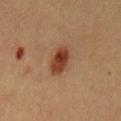biopsy status — imaged on a skin check; not biopsied
patient — female, approximately 40 years of age
image — 15 mm crop, total-body photography
location — the back